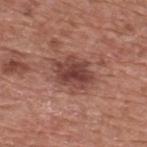patient = male, approximately 75 years of age
image = 15 mm crop, total-body photography
diameter = ~5 mm (longest diameter)
site = the back
tile lighting = white-light illumination